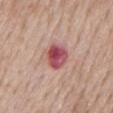Q: Was this lesion biopsied?
A: no biopsy performed (imaged during a skin exam)
Q: How large is the lesion?
A: ≈4 mm
Q: What are the patient's age and sex?
A: male, roughly 75 years of age
Q: Where on the body is the lesion?
A: the mid back
Q: What kind of image is this?
A: ~15 mm crop, total-body skin-cancer survey
Q: What lighting was used for the tile?
A: white-light illumination